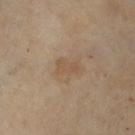- biopsy status: catalogued during a skin exam; not biopsied
- imaging modality: ~15 mm crop, total-body skin-cancer survey
- subject: female, aged approximately 55
- site: the left forearm
- lighting: cross-polarized